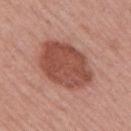Assessment:
Part of a total-body skin-imaging series; this lesion was reviewed on a skin check and was not flagged for biopsy.
Context:
A female patient in their mid-50s. Approximately 7 mm at its widest. Automated image analysis of the tile measured an area of roughly 26 mm², an outline eccentricity of about 0.65 (0 = round, 1 = elongated), and a shape-asymmetry score of about 0.15 (0 = symmetric). It also reported an average lesion color of about L≈49 a*≈26 b*≈28 (CIELAB) and about 13 CIELAB-L* units darker than the surrounding skin. The analysis additionally found a border-irregularity rating of about 1.5/10 and radial color variation of about 1. The analysis additionally found a classifier nevus-likeness of about 80/100 and a lesion-detection confidence of about 100/100. A roughly 15 mm field-of-view crop from a total-body skin photograph. The tile uses white-light illumination. The lesion is located on the arm.